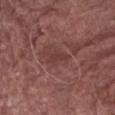No biopsy was performed on this lesion — it was imaged during a full skin examination and was not determined to be concerning. A 15 mm close-up tile from a total-body photography series done for melanoma screening. About 3 mm across. This is a white-light tile. From the abdomen. The subject is a male in their mid- to late 70s.